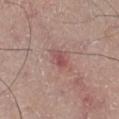This lesion was catalogued during total-body skin photography and was not selected for biopsy.
The tile uses white-light illumination.
The recorded lesion diameter is about 3 mm.
A close-up tile cropped from a whole-body skin photograph, about 15 mm across.
An algorithmic analysis of the crop reported border irregularity of about 2 on a 0–10 scale, a color-variation rating of about 3.5/10, and radial color variation of about 1.5.
A male subject, about 55 years old.